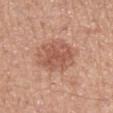Q: Was a biopsy performed?
A: total-body-photography surveillance lesion; no biopsy
Q: What is the anatomic site?
A: the arm
Q: What kind of image is this?
A: 15 mm crop, total-body photography
Q: Who is the patient?
A: female, aged 48–52